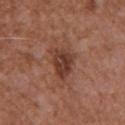Impression: Part of a total-body skin-imaging series; this lesion was reviewed on a skin check and was not flagged for biopsy. Background: A 15 mm close-up extracted from a 3D total-body photography capture. Automated image analysis of the tile measured a footprint of about 9 mm², an eccentricity of roughly 0.7, and a shape-asymmetry score of about 0.35 (0 = symmetric). The analysis additionally found a mean CIELAB color near L≈39 a*≈23 b*≈27, a lesion–skin lightness drop of about 10, and a normalized border contrast of about 8.5. And it measured border irregularity of about 4 on a 0–10 scale, internal color variation of about 3.5 on a 0–10 scale, and a peripheral color-asymmetry measure near 1.5. The patient is a male about 75 years old. On the chest. Longest diameter approximately 4.5 mm. This is a white-light tile.